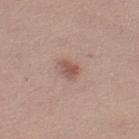The lesion was tiled from a total-body skin photograph and was not biopsied. The patient is a female aged 33–37. Measured at roughly 2.5 mm in maximum diameter. Automated tile analysis of the lesion measured a lesion area of about 3.5 mm², an eccentricity of roughly 0.75, and a shape-asymmetry score of about 0.3 (0 = symmetric). It also reported a mean CIELAB color near L≈53 a*≈20 b*≈25 and about 10 CIELAB-L* units darker than the surrounding skin. And it measured a within-lesion color-variation index near 2/10 and a peripheral color-asymmetry measure near 0.5. The software also gave an automated nevus-likeness rating near 80 out of 100 and lesion-presence confidence of about 100/100. A close-up tile cropped from a whole-body skin photograph, about 15 mm across. From the left thigh. This is a white-light tile.